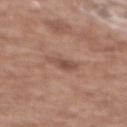  biopsy_status: not biopsied; imaged during a skin examination
  patient:
    sex: female
    age_approx: 75
  image:
    source: total-body photography crop
    field_of_view_mm: 15
  site: upper back